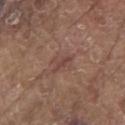A male patient, aged approximately 80. A roughly 15 mm field-of-view crop from a total-body skin photograph. On the left upper arm.